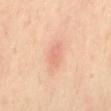Imaged during a routine full-body skin examination; the lesion was not biopsied and no histopathology is available. A female patient in their 40s. Cropped from a whole-body photographic skin survey; the tile spans about 15 mm. The lesion is on the mid back.